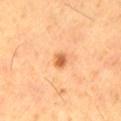Q: Was this lesion biopsied?
A: total-body-photography surveillance lesion; no biopsy
Q: What kind of image is this?
A: total-body-photography crop, ~15 mm field of view
Q: How large is the lesion?
A: ≈2 mm
Q: Patient demographics?
A: male, aged 58–62
Q: What did automated image analysis measure?
A: an average lesion color of about L≈61 a*≈27 b*≈43 (CIELAB); a border-irregularity index near 2/10 and internal color variation of about 3 on a 0–10 scale
Q: How was the tile lit?
A: cross-polarized illumination
Q: Lesion location?
A: the left thigh